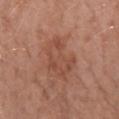Recorded during total-body skin imaging; not selected for excision or biopsy. Captured under white-light illumination. An algorithmic analysis of the crop reported a lesion area of about 14 mm² and a symmetry-axis asymmetry near 0.4. And it measured an average lesion color of about L≈49 a*≈23 b*≈29 (CIELAB) and a lesion–skin lightness drop of about 7. It also reported a border-irregularity index near 4.5/10, internal color variation of about 3 on a 0–10 scale, and peripheral color asymmetry of about 1. The lesion's longest dimension is about 5 mm. The subject is a female approximately 70 years of age. From the arm. A close-up tile cropped from a whole-body skin photograph, about 15 mm across.